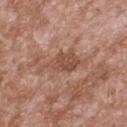A 15 mm close-up tile from a total-body photography series done for melanoma screening.
A male subject, aged 43 to 47.
Located on the upper back.